This image is a 15 mm lesion crop taken from a total-body photograph; Automated image analysis of the tile measured an outline eccentricity of about 0.4 (0 = round, 1 = elongated). The software also gave border irregularity of about 1 on a 0–10 scale and a peripheral color-asymmetry measure near 2.5. And it measured an automated nevus-likeness rating near 15 out of 100 and lesion-presence confidence of about 100/100; from the upper back; the subject is a male roughly 65 years of age; measured at roughly 4 mm in maximum diameter.
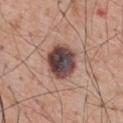Case summary:
– biopsy diagnosis: an atypical melanocytic neoplasm — a lesion of indeterminate malignant potential